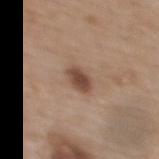Imaged during a routine full-body skin examination; the lesion was not biopsied and no histopathology is available. From the upper back. Longest diameter approximately 2.5 mm. The subject is a female aged 63 to 67. A 15 mm crop from a total-body photograph taken for skin-cancer surveillance. Automated image analysis of the tile measured a footprint of about 4.5 mm² and two-axis asymmetry of about 0.2. It also reported an automated nevus-likeness rating near 90 out of 100 and lesion-presence confidence of about 100/100. Imaged with white-light lighting.